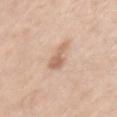  biopsy_status: not biopsied; imaged during a skin examination
  site: left upper arm
  patient:
    sex: male
    age_approx: 60
  automated_metrics:
    area_mm2_approx: 5.0
    eccentricity: 0.9
    shape_asymmetry: 0.4
    vs_skin_darker_L: 11.0
    vs_skin_contrast_norm: 6.5
    nevus_likeness_0_100: 15
    lesion_detection_confidence_0_100: 100
  lesion_size:
    long_diameter_mm_approx: 4.0
  image:
    source: total-body photography crop
    field_of_view_mm: 15